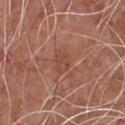Impression:
The lesion was photographed on a routine skin check and not biopsied; there is no pathology result.
Acquisition and patient details:
A male patient, aged 73–77. A 15 mm crop from a total-body photograph taken for skin-cancer surveillance. The lesion is located on the front of the torso. Measured at roughly 2.5 mm in maximum diameter.